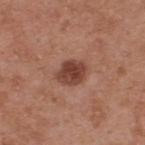No biopsy was performed on this lesion — it was imaged during a full skin examination and was not determined to be concerning. A 15 mm close-up tile from a total-body photography series done for melanoma screening. On the upper back. A male patient aged approximately 55. The recorded lesion diameter is about 3.5 mm. The tile uses white-light illumination. Automated tile analysis of the lesion measured a footprint of about 7.5 mm², an outline eccentricity of about 0.6 (0 = round, 1 = elongated), and two-axis asymmetry of about 0.15. And it measured an average lesion color of about L≈42 a*≈24 b*≈27 (CIELAB) and a lesion–skin lightness drop of about 13. And it measured border irregularity of about 1.5 on a 0–10 scale, internal color variation of about 3.5 on a 0–10 scale, and a peripheral color-asymmetry measure near 1. It also reported a classifier nevus-likeness of about 55/100 and a detector confidence of about 100 out of 100 that the crop contains a lesion.